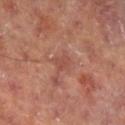{
  "biopsy_status": "not biopsied; imaged during a skin examination",
  "patient": {
    "sex": "male",
    "age_approx": 65
  },
  "site": "leg",
  "image": {
    "source": "total-body photography crop",
    "field_of_view_mm": 15
  }
}